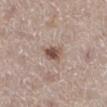{"biopsy_status": "not biopsied; imaged during a skin examination", "lighting": "white-light", "patient": {"sex": "female", "age_approx": 50}, "lesion_size": {"long_diameter_mm_approx": 3.0}, "site": "right lower leg", "image": {"source": "total-body photography crop", "field_of_view_mm": 15}}